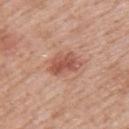follow-up: catalogued during a skin exam; not biopsied
imaging modality: ~15 mm crop, total-body skin-cancer survey
site: the upper back
subject: male, aged around 70
lighting: white-light
image-analysis metrics: a footprint of about 8 mm², an eccentricity of roughly 0.8, and a shape-asymmetry score of about 0.3 (0 = symmetric); a border-irregularity rating of about 3.5/10, a color-variation rating of about 4/10, and radial color variation of about 1.5; an automated nevus-likeness rating near 85 out of 100 and a detector confidence of about 100 out of 100 that the crop contains a lesion